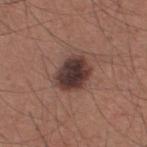The patient is a male roughly 35 years of age. Longest diameter approximately 4 mm. A region of skin cropped from a whole-body photographic capture, roughly 15 mm wide. The lesion is located on the back. The lesion-visualizer software estimated a border-irregularity index near 1.5/10, a color-variation rating of about 5/10, and a peripheral color-asymmetry measure near 1. Imaged with white-light lighting.